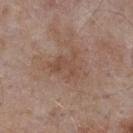biopsy_status: not biopsied; imaged during a skin examination
image:
  source: total-body photography crop
  field_of_view_mm: 15
patient:
  sex: male
  age_approx: 55
lighting: white-light
automated_metrics:
  area_mm2_approx: 7.0
  eccentricity: 0.55
  shape_asymmetry: 0.55
  cielab_L: 48
  cielab_a: 18
  cielab_b: 27
  vs_skin_darker_L: 6.0
  vs_skin_contrast_norm: 5.0
  border_irregularity_0_10: 6.5
  color_variation_0_10: 2.5
  peripheral_color_asymmetry: 0.5
  nevus_likeness_0_100: 0
  lesion_detection_confidence_0_100: 100
site: upper back
lesion_size:
  long_diameter_mm_approx: 3.5